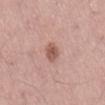On the abdomen.
Automated tile analysis of the lesion measured a lesion color around L≈55 a*≈23 b*≈26 in CIELAB, about 12 CIELAB-L* units darker than the surrounding skin, and a normalized lesion–skin contrast near 8. And it measured a border-irregularity rating of about 2/10 and a peripheral color-asymmetry measure near 1.5. And it measured a classifier nevus-likeness of about 60/100.
A male subject, in their mid-50s.
The recorded lesion diameter is about 3 mm.
A 15 mm crop from a total-body photograph taken for skin-cancer surveillance.
The tile uses white-light illumination.